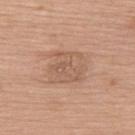Q: How was the tile lit?
A: white-light
Q: Lesion location?
A: the upper back
Q: How large is the lesion?
A: ~5 mm (longest diameter)
Q: What kind of image is this?
A: total-body-photography crop, ~15 mm field of view
Q: What are the patient's age and sex?
A: female, aged around 60